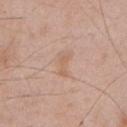• lesion diameter — about 3 mm
• illumination — white-light
• body site — the left upper arm
• patient — male, aged 43 to 47
• acquisition — ~15 mm tile from a whole-body skin photo
• TBP lesion metrics — an area of roughly 4 mm², a shape eccentricity near 0.9, and two-axis asymmetry of about 0.3; border irregularity of about 3.5 on a 0–10 scale, a within-lesion color-variation index near 1/10, and radial color variation of about 0